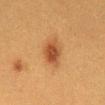<lesion>
  <image>
    <source>total-body photography crop</source>
    <field_of_view_mm>15</field_of_view_mm>
  </image>
  <lighting>cross-polarized</lighting>
  <lesion_size>
    <long_diameter_mm_approx>3.5</long_diameter_mm_approx>
  </lesion_size>
  <automated_metrics>
    <area_mm2_approx>8.0</area_mm2_approx>
    <eccentricity>0.65</eccentricity>
    <shape_asymmetry>0.2</shape_asymmetry>
    <cielab_L>42</cielab_L>
    <cielab_a>22</cielab_a>
    <cielab_b>34</cielab_b>
    <vs_skin_contrast_norm>8.0</vs_skin_contrast_norm>
    <nevus_likeness_0_100>100</nevus_likeness_0_100>
    <lesion_detection_confidence_0_100>100</lesion_detection_confidence_0_100>
  </automated_metrics>
  <patient>
    <sex>female</sex>
    <age_approx>40</age_approx>
  </patient>
  <site>abdomen</site>
</lesion>